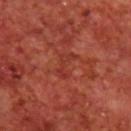Q: Was this lesion biopsied?
A: catalogued during a skin exam; not biopsied
Q: How was the tile lit?
A: cross-polarized
Q: What is the imaging modality?
A: 15 mm crop, total-body photography
Q: What is the lesion's diameter?
A: ≈3.5 mm
Q: What is the anatomic site?
A: the back
Q: Who is the patient?
A: male, aged around 70